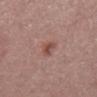Clinical impression: Imaged during a routine full-body skin examination; the lesion was not biopsied and no histopathology is available. Context: A 15 mm crop from a total-body photograph taken for skin-cancer surveillance. From the mid back. A male patient about 55 years old. This is a white-light tile.